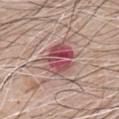Imaged during a routine full-body skin examination; the lesion was not biopsied and no histopathology is available. The lesion is on the chest. The patient is a male aged 68 to 72. A lesion tile, about 15 mm wide, cut from a 3D total-body photograph.